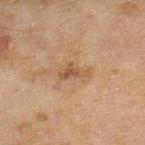The lesion was photographed on a routine skin check and not biopsied; there is no pathology result. Approximately 3.5 mm at its widest. A female subject, aged 58–62. The tile uses cross-polarized illumination. The lesion is located on the right lower leg. Automated tile analysis of the lesion measured a footprint of about 4 mm², an eccentricity of roughly 0.9, and a symmetry-axis asymmetry near 0.5. Cropped from a whole-body photographic skin survey; the tile spans about 15 mm.